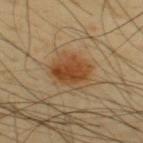{"biopsy_status": "not biopsied; imaged during a skin examination", "patient": {"sex": "male", "age_approx": 45}, "lesion_size": {"long_diameter_mm_approx": 4.5}, "automated_metrics": {"area_mm2_approx": 14.0, "eccentricity": 0.6, "shape_asymmetry": 0.2, "vs_skin_contrast_norm": 8.5}, "image": {"source": "total-body photography crop", "field_of_view_mm": 15}, "site": "upper back"}